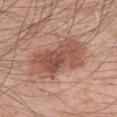<case>
<biopsy_status>not biopsied; imaged during a skin examination</biopsy_status>
<patient>
  <sex>male</sex>
  <age_approx>45</age_approx>
</patient>
<image>
  <source>total-body photography crop</source>
  <field_of_view_mm>15</field_of_view_mm>
</image>
<automated_metrics>
  <shape_asymmetry>0.15</shape_asymmetry>
  <cielab_L>52</cielab_L>
  <cielab_a>22</cielab_a>
  <cielab_b>28</cielab_b>
  <vs_skin_darker_L>11.0</vs_skin_darker_L>
  <vs_skin_contrast_norm>8.0</vs_skin_contrast_norm>
</automated_metrics>
<lesion_size>
  <long_diameter_mm_approx>7.0</long_diameter_mm_approx>
</lesion_size>
<site>left upper arm</site>
</case>